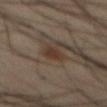notes = imaged on a skin check; not biopsied | tile lighting = cross-polarized illumination | diameter = ≈3 mm | body site = the abdomen | patient = male, in their 60s | image = ~15 mm tile from a whole-body skin photo.